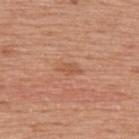Part of a total-body skin-imaging series; this lesion was reviewed on a skin check and was not flagged for biopsy. About 3 mm across. Cropped from a whole-body photographic skin survey; the tile spans about 15 mm. On the upper back. A male subject, in their mid-70s. The lesion-visualizer software estimated a footprint of about 3 mm² and a shape eccentricity near 0.85. Captured under white-light illumination.